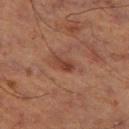Case summary:
• biopsy status: no biopsy performed (imaged during a skin exam)
• imaging modality: total-body-photography crop, ~15 mm field of view
• automated lesion analysis: an automated nevus-likeness rating near 10 out of 100 and a detector confidence of about 100 out of 100 that the crop contains a lesion
• illumination: cross-polarized illumination
• diameter: ≈2.5 mm
• site: the right thigh
• subject: male, aged approximately 65